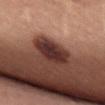Clinical impression: Captured during whole-body skin photography for melanoma surveillance; the lesion was not biopsied. Image and clinical context: A 15 mm close-up extracted from a 3D total-body photography capture. A female subject, aged 18 to 22. Captured under white-light illumination. Longest diameter approximately 5.5 mm. On the upper back. Automated tile analysis of the lesion measured an outline eccentricity of about 0.85 (0 = round, 1 = elongated) and a symmetry-axis asymmetry near 0.2.